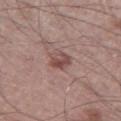{
  "lesion_size": {
    "long_diameter_mm_approx": 2.5
  },
  "lighting": "white-light",
  "automated_metrics": {
    "eccentricity": 0.5,
    "shape_asymmetry": 0.2
  },
  "image": {
    "source": "total-body photography crop",
    "field_of_view_mm": 15
  },
  "patient": {
    "sex": "male",
    "age_approx": 60
  },
  "site": "leg"
}